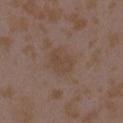Q: Was a biopsy performed?
A: catalogued during a skin exam; not biopsied
Q: Automated lesion metrics?
A: a lesion area of about 8.5 mm², an outline eccentricity of about 0.5 (0 = round, 1 = elongated), and a symmetry-axis asymmetry near 0.15; a lesion color around L≈44 a*≈15 b*≈25 in CIELAB, about 5 CIELAB-L* units darker than the surrounding skin, and a lesion-to-skin contrast of about 5 (normalized; higher = more distinct); internal color variation of about 1.5 on a 0–10 scale and radial color variation of about 0.5; an automated nevus-likeness rating near 0 out of 100 and a detector confidence of about 100 out of 100 that the crop contains a lesion
Q: Who is the patient?
A: female, about 35 years old
Q: Where on the body is the lesion?
A: the left upper arm
Q: What is the lesion's diameter?
A: ≈3.5 mm
Q: What is the imaging modality?
A: 15 mm crop, total-body photography